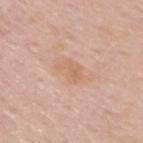Notes:
– notes · no biopsy performed (imaged during a skin exam)
– body site · the upper back
– patient · male, roughly 60 years of age
– image · 15 mm crop, total-body photography